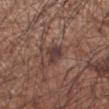Q: Was this lesion biopsied?
A: no biopsy performed (imaged during a skin exam)
Q: Where on the body is the lesion?
A: the left forearm
Q: What kind of image is this?
A: 15 mm crop, total-body photography
Q: Patient demographics?
A: male, aged approximately 55
Q: How large is the lesion?
A: ~2.5 mm (longest diameter)